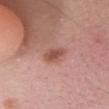Q: Was a biopsy performed?
A: catalogued during a skin exam; not biopsied
Q: Illumination type?
A: white-light
Q: How large is the lesion?
A: about 3 mm
Q: Who is the patient?
A: female, roughly 25 years of age
Q: How was this image acquired?
A: ~15 mm crop, total-body skin-cancer survey
Q: Where on the body is the lesion?
A: the head or neck